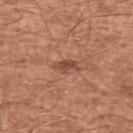Automated image analysis of the tile measured a footprint of about 3.5 mm², an outline eccentricity of about 0.85 (0 = round, 1 = elongated), and a symmetry-axis asymmetry near 0.3. The software also gave a lesion color around L≈48 a*≈23 b*≈28 in CIELAB and about 9 CIELAB-L* units darker than the surrounding skin. The analysis additionally found a border-irregularity rating of about 3/10, internal color variation of about 2 on a 0–10 scale, and radial color variation of about 0.5.
A male subject in their mid-60s.
From the left upper arm.
A lesion tile, about 15 mm wide, cut from a 3D total-body photograph.
The tile uses white-light illumination.